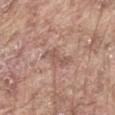Captured during whole-body skin photography for melanoma surveillance; the lesion was not biopsied. A 15 mm crop from a total-body photograph taken for skin-cancer surveillance. Automated tile analysis of the lesion measured a lesion area of about 4.5 mm² and a symmetry-axis asymmetry near 0.5. The analysis additionally found a lesion color around L≈55 a*≈20 b*≈24 in CIELAB, about 8 CIELAB-L* units darker than the surrounding skin, and a lesion-to-skin contrast of about 5.5 (normalized; higher = more distinct). And it measured a within-lesion color-variation index near 1.5/10. The lesion is located on the back. The patient is a male roughly 65 years of age. Imaged with white-light lighting.